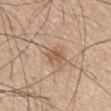workup=total-body-photography surveillance lesion; no biopsy | acquisition=~15 mm tile from a whole-body skin photo | tile lighting=white-light | size=≈3 mm | location=the back | subject=male, aged 63 to 67.